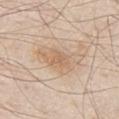Findings:
• follow-up · total-body-photography surveillance lesion; no biopsy
• lighting · white-light illumination
• anatomic site · the front of the torso
• subject · male, aged approximately 80
• image source · ~15 mm tile from a whole-body skin photo
• lesion diameter · ~6.5 mm (longest diameter)